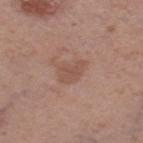No biopsy was performed on this lesion — it was imaged during a full skin examination and was not determined to be concerning.
The lesion is located on the right thigh.
This image is a 15 mm lesion crop taken from a total-body photograph.
A female patient about 55 years old.